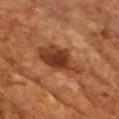{"biopsy_status": "not biopsied; imaged during a skin examination"}